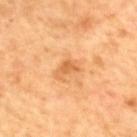Case summary:
• workup · imaged on a skin check; not biopsied
• patient · male, approximately 60 years of age
• location · the upper back
• image source · 15 mm crop, total-body photography